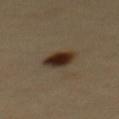follow-up: total-body-photography surveillance lesion; no biopsy | subject: male, aged approximately 40 | site: the back | image source: ~15 mm crop, total-body skin-cancer survey.